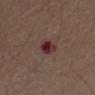| key | value |
|---|---|
| workup | imaged on a skin check; not biopsied |
| acquisition | ~15 mm crop, total-body skin-cancer survey |
| anatomic site | the front of the torso |
| subject | male, aged approximately 70 |
| illumination | white-light |